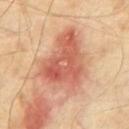Impression: Captured during whole-body skin photography for melanoma surveillance; the lesion was not biopsied.